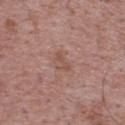Clinical impression:
No biopsy was performed on this lesion — it was imaged during a full skin examination and was not determined to be concerning.
Clinical summary:
A male subject aged 68 to 72. On the upper back. A region of skin cropped from a whole-body photographic capture, roughly 15 mm wide. Measured at roughly 2.5 mm in maximum diameter.